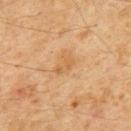Case summary:
* notes · no biopsy performed (imaged during a skin exam)